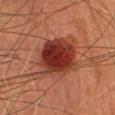{
  "biopsy_status": "not biopsied; imaged during a skin examination",
  "lighting": "cross-polarized",
  "patient": {
    "sex": "female",
    "age_approx": 60
  },
  "site": "head or neck",
  "image": {
    "source": "total-body photography crop",
    "field_of_view_mm": 15
  }
}